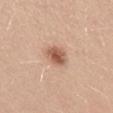Clinical impression:
Captured during whole-body skin photography for melanoma surveillance; the lesion was not biopsied.
Image and clinical context:
A 15 mm close-up extracted from a 3D total-body photography capture. This is a white-light tile. From the mid back. Measured at roughly 3 mm in maximum diameter. Automated tile analysis of the lesion measured an outline eccentricity of about 0.55 (0 = round, 1 = elongated). The analysis additionally found a border-irregularity rating of about 1.5/10 and internal color variation of about 4 on a 0–10 scale. The software also gave lesion-presence confidence of about 100/100. A female patient, aged approximately 25.